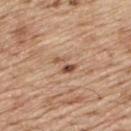Assessment:
Part of a total-body skin-imaging series; this lesion was reviewed on a skin check and was not flagged for biopsy.
Image and clinical context:
A male patient aged approximately 70. Located on the upper back. A 15 mm close-up extracted from a 3D total-body photography capture. The recorded lesion diameter is about 3 mm.